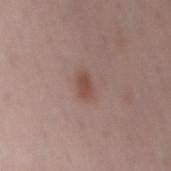This lesion was catalogued during total-body skin photography and was not selected for biopsy.
The lesion is located on the mid back.
A roughly 15 mm field-of-view crop from a total-body skin photograph.
A female patient roughly 50 years of age.